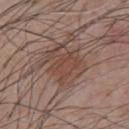Acquisition and patient details:
A male subject, aged around 55. This image is a 15 mm lesion crop taken from a total-body photograph. Located on the chest.